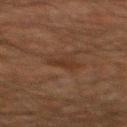follow-up: total-body-photography surveillance lesion; no biopsy | size: ~2.5 mm (longest diameter) | subject: male, aged 58–62 | TBP lesion metrics: a border-irregularity index near 3.5/10, a within-lesion color-variation index near 0.5/10, and radial color variation of about 0 | acquisition: ~15 mm tile from a whole-body skin photo | location: the back.